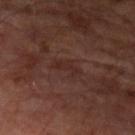Q: Lesion location?
A: the left upper arm
Q: What is the imaging modality?
A: 15 mm crop, total-body photography
Q: Automated lesion metrics?
A: a shape eccentricity near 0.9 and two-axis asymmetry of about 0.55; an average lesion color of about L≈26 a*≈18 b*≈20 (CIELAB) and a lesion–skin lightness drop of about 5; a classifier nevus-likeness of about 0/100 and a lesion-detection confidence of about 75/100
Q: What are the patient's age and sex?
A: male, aged approximately 65